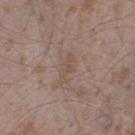Captured during whole-body skin photography for melanoma surveillance; the lesion was not biopsied.
The lesion's longest dimension is about 3.5 mm.
The tile uses white-light illumination.
A male subject approximately 45 years of age.
A 15 mm close-up extracted from a 3D total-body photography capture.
Located on the right thigh.